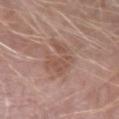<lesion>
<biopsy_status>not biopsied; imaged during a skin examination</biopsy_status>
<patient>
  <sex>male</sex>
  <age_approx>50</age_approx>
</patient>
<site>left forearm</site>
<lighting>white-light</lighting>
<image>
  <source>total-body photography crop</source>
  <field_of_view_mm>15</field_of_view_mm>
</image>
<automated_metrics>
  <cielab_L>52</cielab_L>
  <cielab_a>19</cielab_a>
  <cielab_b>26</cielab_b>
  <vs_skin_darker_L>8.0</vs_skin_darker_L>
  <border_irregularity_0_10>8.0</border_irregularity_0_10>
  <color_variation_0_10>2.5</color_variation_0_10>
  <peripheral_color_asymmetry>1.0</peripheral_color_asymmetry>
  <lesion_detection_confidence_0_100>100</lesion_detection_confidence_0_100>
</automated_metrics>
<lesion_size>
  <long_diameter_mm_approx>5.0</long_diameter_mm_approx>
</lesion_size>
</lesion>